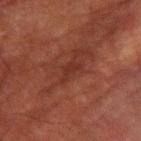{
  "biopsy_status": "not biopsied; imaged during a skin examination",
  "site": "arm",
  "patient": {
    "sex": "male",
    "age_approx": 80
  },
  "automated_metrics": {
    "cielab_L": 25,
    "cielab_a": 22,
    "cielab_b": 22,
    "vs_skin_darker_L": 5.0,
    "vs_skin_contrast_norm": 5.5,
    "color_variation_0_10": 1.0,
    "peripheral_color_asymmetry": 0.5
  },
  "image": {
    "source": "total-body photography crop",
    "field_of_view_mm": 15
  },
  "lesion_size": {
    "long_diameter_mm_approx": 3.0
  }
}